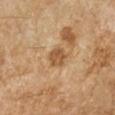Assessment: Captured during whole-body skin photography for melanoma surveillance; the lesion was not biopsied. Clinical summary: The total-body-photography lesion software estimated an area of roughly 5 mm², an outline eccentricity of about 0.7 (0 = round, 1 = elongated), and a shape-asymmetry score of about 0.2 (0 = symmetric). The software also gave a lesion color around L≈55 a*≈20 b*≈38 in CIELAB, about 11 CIELAB-L* units darker than the surrounding skin, and a normalized lesion–skin contrast near 7.5. This is a cross-polarized tile. On the arm. The lesion's longest dimension is about 3 mm. Cropped from a whole-body photographic skin survey; the tile spans about 15 mm. A female patient, aged 68 to 72.